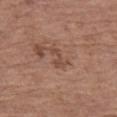Background:
Automated image analysis of the tile measured a border-irregularity index near 7.5/10, a within-lesion color-variation index near 0/10, and a peripheral color-asymmetry measure near 0. The software also gave a classifier nevus-likeness of about 0/100 and lesion-presence confidence of about 100/100. A female patient, aged around 65. The lesion is on the leg. A lesion tile, about 15 mm wide, cut from a 3D total-body photograph.